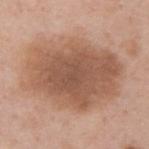notes: imaged on a skin check; not biopsied
automated lesion analysis: a footprint of about 55 mm², an outline eccentricity of about 0.7 (0 = round, 1 = elongated), and two-axis asymmetry of about 0.15; a lesion color around L≈55 a*≈20 b*≈30 in CIELAB and a lesion-to-skin contrast of about 8 (normalized; higher = more distinct); an automated nevus-likeness rating near 25 out of 100
patient: male, about 40 years old
site: the upper back
image: total-body-photography crop, ~15 mm field of view